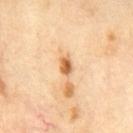Part of a total-body skin-imaging series; this lesion was reviewed on a skin check and was not flagged for biopsy. This image is a 15 mm lesion crop taken from a total-body photograph. The tile uses cross-polarized illumination. The lesion is located on the chest. The patient is a male aged 68–72.